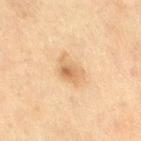Part of a total-body skin-imaging series; this lesion was reviewed on a skin check and was not flagged for biopsy. A 15 mm close-up tile from a total-body photography series done for melanoma screening. The recorded lesion diameter is about 4 mm. This is a cross-polarized tile. A female patient, in their mid-50s. The lesion is located on the right thigh.